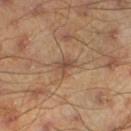This lesion was catalogued during total-body skin photography and was not selected for biopsy.
A male subject, approximately 45 years of age.
This image is a 15 mm lesion crop taken from a total-body photograph.
From the left lower leg.